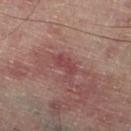workup = imaged on a skin check; not biopsied
acquisition = ~15 mm crop, total-body skin-cancer survey
patient = male, approximately 60 years of age
automated lesion analysis = an automated nevus-likeness rating near 0 out of 100
body site = the right lower leg